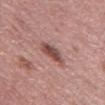Part of a total-body skin-imaging series; this lesion was reviewed on a skin check and was not flagged for biopsy.